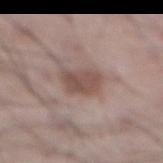{
  "biopsy_status": "not biopsied; imaged during a skin examination",
  "image": {
    "source": "total-body photography crop",
    "field_of_view_mm": 15
  },
  "site": "front of the torso",
  "automated_metrics": {
    "area_mm2_approx": 7.5,
    "eccentricity": 0.6,
    "shape_asymmetry": 0.25,
    "cielab_L": 49,
    "cielab_a": 17,
    "cielab_b": 22,
    "vs_skin_darker_L": 10.0,
    "vs_skin_contrast_norm": 7.5,
    "lesion_detection_confidence_0_100": 100
  },
  "patient": {
    "sex": "male",
    "age_approx": 55
  },
  "lighting": "white-light"
}